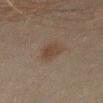Q: Was a biopsy performed?
A: imaged on a skin check; not biopsied
Q: What is the lesion's diameter?
A: ~3 mm (longest diameter)
Q: What did automated image analysis measure?
A: a lesion area of about 5.5 mm² and an outline eccentricity of about 0.6 (0 = round, 1 = elongated); an average lesion color of about L≈33 a*≈12 b*≈22 (CIELAB), about 6 CIELAB-L* units darker than the surrounding skin, and a normalized lesion–skin contrast near 6.5; a border-irregularity index near 2.5/10, internal color variation of about 2 on a 0–10 scale, and peripheral color asymmetry of about 0.5
Q: How was this image acquired?
A: total-body-photography crop, ~15 mm field of view
Q: What are the patient's age and sex?
A: male, aged 43 to 47
Q: What lighting was used for the tile?
A: cross-polarized illumination
Q: What is the anatomic site?
A: the front of the torso